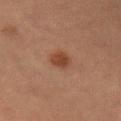The lesion was photographed on a routine skin check and not biopsied; there is no pathology result.
On the right upper arm.
The lesion's longest dimension is about 2.5 mm.
The total-body-photography lesion software estimated a lesion area of about 4.5 mm² and a symmetry-axis asymmetry near 0.2. The software also gave a mean CIELAB color near L≈33 a*≈19 b*≈26, about 8 CIELAB-L* units darker than the surrounding skin, and a normalized lesion–skin contrast near 8. The software also gave a border-irregularity rating of about 1.5/10 and internal color variation of about 1.5 on a 0–10 scale.
This is a cross-polarized tile.
A male patient in their mid-60s.
A roughly 15 mm field-of-view crop from a total-body skin photograph.